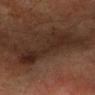{
  "biopsy_status": "not biopsied; imaged during a skin examination",
  "image": {
    "source": "total-body photography crop",
    "field_of_view_mm": 15
  },
  "patient": {
    "sex": "male",
    "age_approx": 60
  },
  "site": "left forearm",
  "lighting": "cross-polarized",
  "lesion_size": {
    "long_diameter_mm_approx": 9.0
  }
}